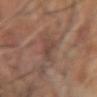Notes:
- follow-up: no biopsy performed (imaged during a skin exam)
- acquisition: total-body-photography crop, ~15 mm field of view
- location: the left forearm
- patient: male, aged around 65
- tile lighting: cross-polarized
- diameter: ~4.5 mm (longest diameter)